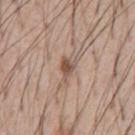{"biopsy_status": "not biopsied; imaged during a skin examination", "lesion_size": {"long_diameter_mm_approx": 2.5}, "site": "abdomen", "image": {"source": "total-body photography crop", "field_of_view_mm": 15}, "patient": {"sex": "male", "age_approx": 55}, "lighting": "white-light"}